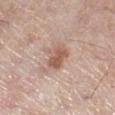{"biopsy_status": "not biopsied; imaged during a skin examination", "lighting": "white-light", "image": {"source": "total-body photography crop", "field_of_view_mm": 15}, "patient": {"sex": "male", "age_approx": 70}, "site": "leg"}